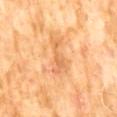No biopsy was performed on this lesion — it was imaged during a full skin examination and was not determined to be concerning.
A male subject, roughly 60 years of age.
This is a cross-polarized tile.
A 15 mm close-up tile from a total-body photography series done for melanoma screening.
On the abdomen.